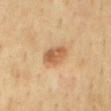follow-up=catalogued during a skin exam; not biopsied | illumination=cross-polarized illumination | diameter=~3.5 mm (longest diameter) | subject=male, about 70 years old | TBP lesion metrics=an area of roughly 7 mm², a shape eccentricity near 0.75, and a symmetry-axis asymmetry near 0.15; a classifier nevus-likeness of about 85/100 and lesion-presence confidence of about 100/100 | image source=15 mm crop, total-body photography | location=the mid back.